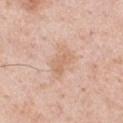workup: catalogued during a skin exam; not biopsied
diameter: ≈4.5 mm
site: the chest
patient: male, approximately 50 years of age
acquisition: ~15 mm crop, total-body skin-cancer survey
automated metrics: a lesion color around L≈67 a*≈19 b*≈31 in CIELAB, a lesion–skin lightness drop of about 7, and a lesion-to-skin contrast of about 5 (normalized; higher = more distinct)